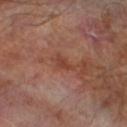On the left lower leg. A 15 mm close-up extracted from a 3D total-body photography capture. The total-body-photography lesion software estimated a lesion color around L≈40 a*≈23 b*≈28 in CIELAB, a lesion–skin lightness drop of about 7, and a lesion-to-skin contrast of about 6 (normalized; higher = more distinct). And it measured a border-irregularity index near 4/10, a color-variation rating of about 1/10, and a peripheral color-asymmetry measure near 0. And it measured a detector confidence of about 100 out of 100 that the crop contains a lesion. Approximately 2.5 mm at its widest. A male subject aged around 70.